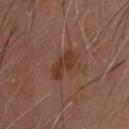Q: How was the tile lit?
A: cross-polarized illumination
Q: Who is the patient?
A: male, in their mid- to late 60s
Q: How was this image acquired?
A: ~15 mm tile from a whole-body skin photo
Q: Where on the body is the lesion?
A: the head or neck
Q: What is the lesion's diameter?
A: about 4 mm